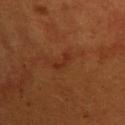The lesion's longest dimension is about 2.5 mm. The patient is a female aged approximately 30. From the upper back. A lesion tile, about 15 mm wide, cut from a 3D total-body photograph. The lesion-visualizer software estimated an area of roughly 2.5 mm², an eccentricity of roughly 0.9, and a symmetry-axis asymmetry near 0.55. Imaged with cross-polarized lighting.